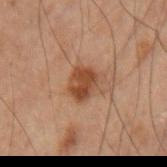This lesion was catalogued during total-body skin photography and was not selected for biopsy. A close-up tile cropped from a whole-body skin photograph, about 15 mm across. The total-body-photography lesion software estimated an average lesion color of about L≈34 a*≈19 b*≈27 (CIELAB), about 9 CIELAB-L* units darker than the surrounding skin, and a normalized border contrast of about 9. It also reported peripheral color asymmetry of about 1. The tile uses cross-polarized illumination. Located on the left upper arm. About 3 mm across. The subject is a male aged 68 to 72.